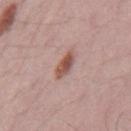Q: Was this lesion biopsied?
A: total-body-photography surveillance lesion; no biopsy
Q: What kind of image is this?
A: total-body-photography crop, ~15 mm field of view
Q: What did automated image analysis measure?
A: a color-variation rating of about 4/10 and a peripheral color-asymmetry measure near 1.5; an automated nevus-likeness rating near 90 out of 100 and lesion-presence confidence of about 100/100
Q: How large is the lesion?
A: ≈3 mm
Q: What is the anatomic site?
A: the mid back
Q: Patient demographics?
A: male, aged around 70